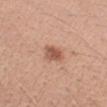Impression: This lesion was catalogued during total-body skin photography and was not selected for biopsy. Background: Located on the arm. The lesion's longest dimension is about 3 mm. Imaged with white-light lighting. The lesion-visualizer software estimated a mean CIELAB color near L≈55 a*≈23 b*≈31 and a normalized lesion–skin contrast near 8. It also reported border irregularity of about 2.5 on a 0–10 scale, a color-variation rating of about 3/10, and peripheral color asymmetry of about 1. A 15 mm crop from a total-body photograph taken for skin-cancer surveillance. A female patient, approximately 45 years of age.